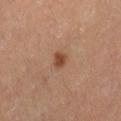* notes · no biopsy performed (imaged during a skin exam)
* tile lighting · cross-polarized
* image · ~15 mm tile from a whole-body skin photo
* patient · female, roughly 55 years of age
* automated metrics · a lesion color around L≈42 a*≈21 b*≈30 in CIELAB, about 10 CIELAB-L* units darker than the surrounding skin, and a normalized lesion–skin contrast near 8.5; a border-irregularity rating of about 2/10 and internal color variation of about 1.5 on a 0–10 scale
* anatomic site · the leg
* lesion diameter · ≈2 mm